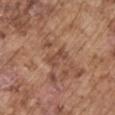{"biopsy_status": "not biopsied; imaged during a skin examination", "image": {"source": "total-body photography crop", "field_of_view_mm": 15}, "patient": {"sex": "male", "age_approx": 75}, "site": "right upper arm", "lesion_size": {"long_diameter_mm_approx": 3.0}, "automated_metrics": {"cielab_L": 47, "cielab_a": 21, "cielab_b": 28, "vs_skin_darker_L": 8.0, "vs_skin_contrast_norm": 6.0, "nevus_likeness_0_100": 0, "lesion_detection_confidence_0_100": 95}}